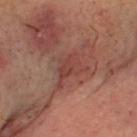Case summary:
– workup — catalogued during a skin exam; not biopsied
– subject — male, aged around 55
– lesion size — ≈4 mm
– acquisition — ~15 mm tile from a whole-body skin photo
– automated metrics — a border-irregularity rating of about 6.5/10, a color-variation rating of about 2.5/10, and peripheral color asymmetry of about 1; an automated nevus-likeness rating near 0 out of 100
– body site — the head or neck